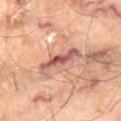Q: Was this lesion biopsied?
A: total-body-photography surveillance lesion; no biopsy
Q: What is the imaging modality?
A: ~15 mm crop, total-body skin-cancer survey
Q: What did automated image analysis measure?
A: a footprint of about 8 mm², a shape eccentricity near 0.95, and a shape-asymmetry score of about 0.25 (0 = symmetric); border irregularity of about 4.5 on a 0–10 scale and a peripheral color-asymmetry measure near 2; a lesion-detection confidence of about 80/100
Q: Where on the body is the lesion?
A: the right thigh
Q: Patient demographics?
A: male, in their 70s
Q: Illumination type?
A: cross-polarized